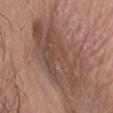Assessment: Imaged during a routine full-body skin examination; the lesion was not biopsied and no histopathology is available. Image and clinical context: Automated image analysis of the tile measured an outline eccentricity of about 0.85 (0 = round, 1 = elongated). The analysis additionally found a border-irregularity rating of about 7.5/10, a color-variation rating of about 4.5/10, and radial color variation of about 1.5. And it measured a nevus-likeness score of about 0/100 and lesion-presence confidence of about 85/100. A close-up tile cropped from a whole-body skin photograph, about 15 mm across. The lesion is located on the head or neck. Longest diameter approximately 8.5 mm. The tile uses white-light illumination. A male patient, about 60 years old.